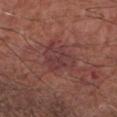Notes:
• follow-up · no biopsy performed (imaged during a skin exam)
• automated metrics · a detector confidence of about 100 out of 100 that the crop contains a lesion
• illumination · white-light
• subject · male, about 65 years old
• lesion size · ≈5.5 mm
• image · total-body-photography crop, ~15 mm field of view
• anatomic site · the right forearm